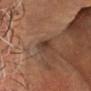This lesion was catalogued during total-body skin photography and was not selected for biopsy. A male patient in their 60s. From the head or neck. A close-up tile cropped from a whole-body skin photograph, about 15 mm across. Imaged with cross-polarized lighting.